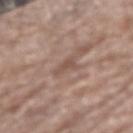Case summary:
- follow-up — total-body-photography surveillance lesion; no biopsy
- subject — male, about 70 years old
- acquisition — ~15 mm tile from a whole-body skin photo
- tile lighting — white-light illumination
- image-analysis metrics — a footprint of about 3 mm² and a shape-asymmetry score of about 0.4 (0 = symmetric); an average lesion color of about L≈51 a*≈17 b*≈25 (CIELAB), a lesion–skin lightness drop of about 8, and a lesion-to-skin contrast of about 6 (normalized; higher = more distinct); a nevus-likeness score of about 0/100 and a detector confidence of about 80 out of 100 that the crop contains a lesion
- lesion size — about 2.5 mm
- anatomic site — the arm